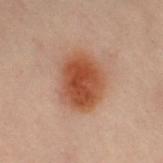Impression: No biopsy was performed on this lesion — it was imaged during a full skin examination and was not determined to be concerning. Acquisition and patient details: A region of skin cropped from a whole-body photographic capture, roughly 15 mm wide. On the right leg. A female patient aged 58 to 62.